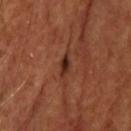No biopsy was performed on this lesion — it was imaged during a full skin examination and was not determined to be concerning. Imaged with cross-polarized lighting. Measured at roughly 3 mm in maximum diameter. On the head or neck. A 15 mm crop from a total-body photograph taken for skin-cancer surveillance.